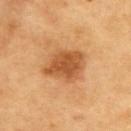Clinical impression: No biopsy was performed on this lesion — it was imaged during a full skin examination and was not determined to be concerning. Image and clinical context: A 15 mm close-up tile from a total-body photography series done for melanoma screening. A male patient, aged 58 to 62. The lesion is located on the upper back.